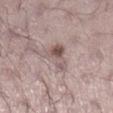follow-up: catalogued during a skin exam; not biopsied
tile lighting: white-light illumination
image: total-body-photography crop, ~15 mm field of view
subject: male, about 25 years old
anatomic site: the right lower leg
lesion size: ≈4.5 mm